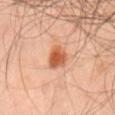biopsy status: imaged on a skin check; not biopsied
TBP lesion metrics: a lesion area of about 7 mm² and a shape eccentricity near 0.55; a lesion–skin lightness drop of about 13 and a normalized lesion–skin contrast near 9; a nevus-likeness score of about 100/100 and lesion-presence confidence of about 100/100
image source: ~15 mm tile from a whole-body skin photo
patient: male, approximately 45 years of age
lesion diameter: ≈3 mm
body site: the mid back
illumination: cross-polarized